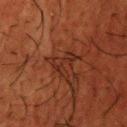The lesion was photographed on a routine skin check and not biopsied; there is no pathology result. This is a cross-polarized tile. From the head or neck. A male patient, approximately 60 years of age. A 15 mm close-up tile from a total-body photography series done for melanoma screening. Approximately 3.5 mm at its widest.